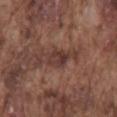The lesion was tiled from a total-body skin photograph and was not biopsied. A male patient about 75 years old. The lesion is located on the mid back. An algorithmic analysis of the crop reported a footprint of about 5.5 mm², an outline eccentricity of about 0.65 (0 = round, 1 = elongated), and a symmetry-axis asymmetry near 0.25. The software also gave border irregularity of about 2.5 on a 0–10 scale, internal color variation of about 4.5 on a 0–10 scale, and radial color variation of about 1.5. A 15 mm crop from a total-body photograph taken for skin-cancer surveillance. About 3 mm across.